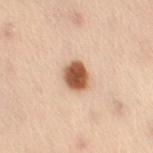Clinical impression: No biopsy was performed on this lesion — it was imaged during a full skin examination and was not determined to be concerning. Context: Automated image analysis of the tile measured a shape-asymmetry score of about 0.2 (0 = symmetric). And it measured a lesion-to-skin contrast of about 13 (normalized; higher = more distinct). A female subject aged approximately 50. Located on the right thigh. A close-up tile cropped from a whole-body skin photograph, about 15 mm across. Longest diameter approximately 3.5 mm.